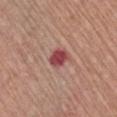Captured during whole-body skin photography for melanoma surveillance; the lesion was not biopsied.
The lesion is on the right upper arm.
Captured under white-light illumination.
Longest diameter approximately 2.5 mm.
A male subject approximately 65 years of age.
A region of skin cropped from a whole-body photographic capture, roughly 15 mm wide.
Automated tile analysis of the lesion measured a lesion color around L≈47 a*≈31 b*≈22 in CIELAB, about 14 CIELAB-L* units darker than the surrounding skin, and a normalized border contrast of about 10.5. The software also gave a border-irregularity rating of about 2/10, a within-lesion color-variation index near 3/10, and radial color variation of about 1. It also reported a classifier nevus-likeness of about 0/100 and a lesion-detection confidence of about 100/100.